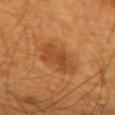Assessment:
Recorded during total-body skin imaging; not selected for excision or biopsy.
Clinical summary:
The lesion is located on the left upper arm. The patient is a male roughly 60 years of age. Automated image analysis of the tile measured a lesion area of about 10 mm², a shape eccentricity near 0.6, and a symmetry-axis asymmetry near 0.3. And it measured a lesion color around L≈46 a*≈26 b*≈41 in CIELAB, roughly 8 lightness units darker than nearby skin, and a normalized lesion–skin contrast near 6. And it measured internal color variation of about 3 on a 0–10 scale and peripheral color asymmetry of about 1. A 15 mm close-up tile from a total-body photography series done for melanoma screening. Imaged with cross-polarized lighting.